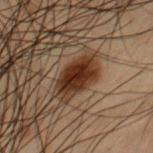<record>
  <biopsy_status>not biopsied; imaged during a skin examination</biopsy_status>
  <automated_metrics>
    <cielab_L>24</cielab_L>
    <cielab_a>17</cielab_a>
    <cielab_b>24</cielab_b>
    <vs_skin_contrast_norm>14.0</vs_skin_contrast_norm>
    <border_irregularity_0_10>1.5</border_irregularity_0_10>
    <color_variation_0_10>4.0</color_variation_0_10>
    <peripheral_color_asymmetry>1.0</peripheral_color_asymmetry>
  </automated_metrics>
  <site>front of the torso</site>
  <patient>
    <sex>male</sex>
    <age_approx>55</age_approx>
  </patient>
  <image>
    <source>total-body photography crop</source>
    <field_of_view_mm>15</field_of_view_mm>
  </image>
</record>